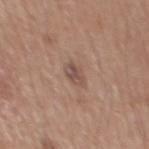Q: Is there a histopathology result?
A: imaged on a skin check; not biopsied
Q: What did automated image analysis measure?
A: a lesion area of about 3.5 mm², a shape eccentricity near 0.7, and two-axis asymmetry of about 0.3; a mean CIELAB color near L≈50 a*≈18 b*≈24, a lesion–skin lightness drop of about 9, and a lesion-to-skin contrast of about 6.5 (normalized; higher = more distinct); a nevus-likeness score of about 5/100
Q: Illumination type?
A: white-light
Q: How was this image acquired?
A: total-body-photography crop, ~15 mm field of view
Q: What is the anatomic site?
A: the mid back
Q: How large is the lesion?
A: ~2.5 mm (longest diameter)
Q: Who is the patient?
A: male, aged approximately 65